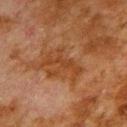The lesion was tiled from a total-body skin photograph and was not biopsied. A close-up tile cropped from a whole-body skin photograph, about 15 mm across. From the upper back. A male patient, aged 78–82. The tile uses cross-polarized illumination. The recorded lesion diameter is about 5 mm.